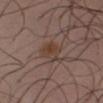Clinical summary: Measured at roughly 3 mm in maximum diameter. The tile uses white-light illumination. The patient is a male aged around 40. The lesion is on the front of the torso. Cropped from a whole-body photographic skin survey; the tile spans about 15 mm.